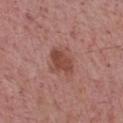biopsy status = no biopsy performed (imaged during a skin exam); location = the chest; subject = male, roughly 75 years of age; illumination = white-light illumination; image = 15 mm crop, total-body photography.